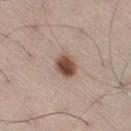notes: catalogued during a skin exam; not biopsied | imaging modality: total-body-photography crop, ~15 mm field of view | automated lesion analysis: two-axis asymmetry of about 0.1; a mean CIELAB color near L≈48 a*≈19 b*≈26, about 17 CIELAB-L* units darker than the surrounding skin, and a normalized border contrast of about 11.5 | subject: male, about 55 years old | diameter: ~3 mm (longest diameter) | site: the right thigh | lighting: white-light.